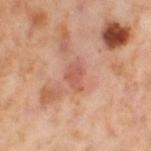Q: Was a biopsy performed?
A: total-body-photography surveillance lesion; no biopsy
Q: What is the imaging modality?
A: total-body-photography crop, ~15 mm field of view
Q: Patient demographics?
A: female, aged around 55
Q: What lighting was used for the tile?
A: cross-polarized
Q: Lesion location?
A: the right thigh
Q: What did automated image analysis measure?
A: a nevus-likeness score of about 0/100 and a lesion-detection confidence of about 100/100